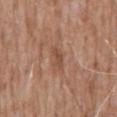Assessment:
Captured during whole-body skin photography for melanoma surveillance; the lesion was not biopsied.
Acquisition and patient details:
A 15 mm close-up tile from a total-body photography series done for melanoma screening. Measured at roughly 2.5 mm in maximum diameter. The lesion is located on the abdomen. A male patient aged 58 to 62. This is a white-light tile.